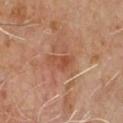biopsy_status: not biopsied; imaged during a skin examination